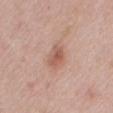<tbp_lesion>
  <biopsy_status>not biopsied; imaged during a skin examination</biopsy_status>
  <image>
    <source>total-body photography crop</source>
    <field_of_view_mm>15</field_of_view_mm>
  </image>
  <patient>
    <sex>female</sex>
    <age_approx>40</age_approx>
  </patient>
  <lesion_size>
    <long_diameter_mm_approx>3.0</long_diameter_mm_approx>
  </lesion_size>
  <site>mid back</site>
</tbp_lesion>